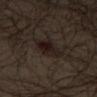workup: total-body-photography surveillance lesion; no biopsy | body site: the left thigh | acquisition: total-body-photography crop, ~15 mm field of view | subject: male, roughly 65 years of age.